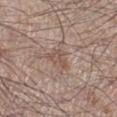<case>
  <biopsy_status>not biopsied; imaged during a skin examination</biopsy_status>
  <patient>
    <sex>male</sex>
    <age_approx>55</age_approx>
  </patient>
  <site>right lower leg</site>
  <image>
    <source>total-body photography crop</source>
    <field_of_view_mm>15</field_of_view_mm>
  </image>
</case>